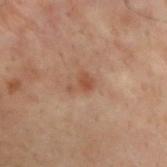<lesion>
<biopsy_status>not biopsied; imaged during a skin examination</biopsy_status>
<lighting>cross-polarized</lighting>
<automated_metrics>
  <area_mm2_approx>3.0</area_mm2_approx>
  <cielab_L>40</cielab_L>
  <cielab_a>18</cielab_a>
  <cielab_b>26</cielab_b>
  <vs_skin_darker_L>7.0</vs_skin_darker_L>
  <vs_skin_contrast_norm>6.5</vs_skin_contrast_norm>
  <border_irregularity_0_10>6.5</border_irregularity_0_10>
  <color_variation_0_10>1.5</color_variation_0_10>
  <peripheral_color_asymmetry>0.5</peripheral_color_asymmetry>
</automated_metrics>
<image>
  <source>total-body photography crop</source>
  <field_of_view_mm>15</field_of_view_mm>
</image>
<lesion_size>
  <long_diameter_mm_approx>2.5</long_diameter_mm_approx>
</lesion_size>
<site>upper back</site>
<patient>
  <sex>male</sex>
  <age_approx>30</age_approx>
</patient>
</lesion>